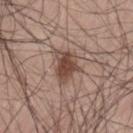Notes:
• biopsy status · no biopsy performed (imaged during a skin exam)
• location · the lower back
• patient · male, aged 68 to 72
• illumination · white-light illumination
• acquisition · 15 mm crop, total-body photography
• diameter · ≈4 mm
• automated lesion analysis · a lesion color around L≈46 a*≈18 b*≈25 in CIELAB, roughly 12 lightness units darker than nearby skin, and a normalized lesion–skin contrast near 9; a classifier nevus-likeness of about 95/100 and a lesion-detection confidence of about 100/100